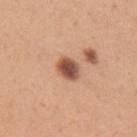notes — total-body-photography surveillance lesion; no biopsy | image — total-body-photography crop, ~15 mm field of view | anatomic site — the right upper arm | patient — female, about 30 years old.